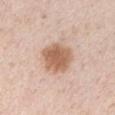This image is a 15 mm lesion crop taken from a total-body photograph. A male subject, approximately 40 years of age. On the arm. The tile uses white-light illumination. The lesion's longest dimension is about 4 mm.